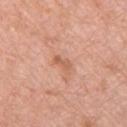| field | value |
|---|---|
| biopsy status | total-body-photography surveillance lesion; no biopsy |
| imaging modality | ~15 mm tile from a whole-body skin photo |
| lesion diameter | ~2.5 mm (longest diameter) |
| site | the chest |
| illumination | white-light |
| subject | male, aged 53 to 57 |